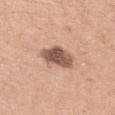This lesion was catalogued during total-body skin photography and was not selected for biopsy.
A female patient aged around 35.
The lesion is on the left upper arm.
A 15 mm close-up extracted from a 3D total-body photography capture.